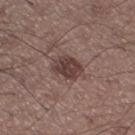{"biopsy_status": "not biopsied; imaged during a skin examination", "site": "right thigh", "lighting": "white-light", "patient": {"sex": "male", "age_approx": 55}, "lesion_size": {"long_diameter_mm_approx": 3.5}, "image": {"source": "total-body photography crop", "field_of_view_mm": 15}}